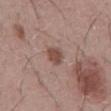Recorded during total-body skin imaging; not selected for excision or biopsy. A male subject about 45 years old. On the abdomen. A 15 mm close-up tile from a total-body photography series done for melanoma screening.